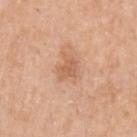Clinical impression:
This lesion was catalogued during total-body skin photography and was not selected for biopsy.
Background:
The tile uses white-light illumination. The lesion-visualizer software estimated an area of roughly 4.5 mm², a shape eccentricity near 0.7, and a symmetry-axis asymmetry near 0.35. It also reported a lesion color around L≈61 a*≈22 b*≈35 in CIELAB, about 8 CIELAB-L* units darker than the surrounding skin, and a lesion-to-skin contrast of about 6 (normalized; higher = more distinct). And it measured a classifier nevus-likeness of about 0/100 and lesion-presence confidence of about 100/100. The lesion is on the left upper arm. A female subject aged 58 to 62. A 15 mm crop from a total-body photograph taken for skin-cancer surveillance. About 3 mm across.